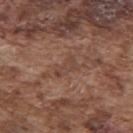| field | value |
|---|---|
| follow-up | no biopsy performed (imaged during a skin exam) |
| imaging modality | ~15 mm tile from a whole-body skin photo |
| subject | male, aged approximately 75 |
| site | the upper back |
| illumination | white-light illumination |